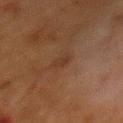- notes · total-body-photography surveillance lesion; no biopsy
- body site · the mid back
- image · total-body-photography crop, ~15 mm field of view
- automated metrics · a mean CIELAB color near L≈29 a*≈16 b*≈25, roughly 5 lightness units darker than nearby skin, and a lesion-to-skin contrast of about 5.5 (normalized; higher = more distinct)
- subject · female, roughly 50 years of age
- illumination · cross-polarized
- diameter · ~2.5 mm (longest diameter)